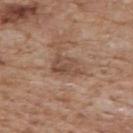image source — ~15 mm crop, total-body skin-cancer survey
tile lighting — white-light
anatomic site — the chest
patient — male, aged 58 to 62
size — ≈4 mm
automated metrics — an area of roughly 7.5 mm² and a shape-asymmetry score of about 0.3 (0 = symmetric); a lesion color around L≈49 a*≈18 b*≈28 in CIELAB, a lesion–skin lightness drop of about 9, and a normalized lesion–skin contrast near 6.5; a border-irregularity index near 3.5/10 and a color-variation rating of about 4/10; a classifier nevus-likeness of about 0/100 and a detector confidence of about 100 out of 100 that the crop contains a lesion